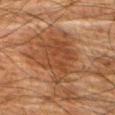Q: Is there a histopathology result?
A: catalogued during a skin exam; not biopsied
Q: How was this image acquired?
A: ~15 mm tile from a whole-body skin photo
Q: What are the patient's age and sex?
A: male, about 80 years old
Q: Lesion location?
A: the front of the torso